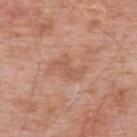<lesion>
  <biopsy_status>not biopsied; imaged during a skin examination</biopsy_status>
  <patient>
    <sex>male</sex>
    <age_approx>60</age_approx>
  </patient>
  <image>
    <source>total-body photography crop</source>
    <field_of_view_mm>15</field_of_view_mm>
  </image>
  <site>upper back</site>
</lesion>